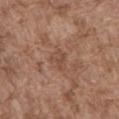Impression:
Captured during whole-body skin photography for melanoma surveillance; the lesion was not biopsied.
Background:
Measured at roughly 2.5 mm in maximum diameter. Cropped from a whole-body photographic skin survey; the tile spans about 15 mm. Located on the front of the torso. This is a white-light tile. A male patient, approximately 75 years of age.